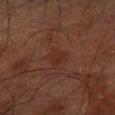Imaged during a routine full-body skin examination; the lesion was not biopsied and no histopathology is available. This is a cross-polarized tile. Automated tile analysis of the lesion measured an average lesion color of about L≈21 a*≈17 b*≈22 (CIELAB), a lesion–skin lightness drop of about 4, and a normalized border contrast of about 5.5. The software also gave border irregularity of about 3 on a 0–10 scale, internal color variation of about 1 on a 0–10 scale, and a peripheral color-asymmetry measure near 0.5. And it measured a nevus-likeness score of about 0/100 and lesion-presence confidence of about 100/100. A lesion tile, about 15 mm wide, cut from a 3D total-body photograph. From the left forearm. Measured at roughly 3 mm in maximum diameter. A male patient, about 60 years old.